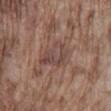follow-up=no biopsy performed (imaged during a skin exam); imaging modality=total-body-photography crop, ~15 mm field of view; site=the mid back; patient=male, in their mid-70s.